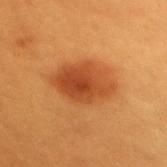Clinical impression:
The lesion was photographed on a routine skin check and not biopsied; there is no pathology result.
Background:
A lesion tile, about 15 mm wide, cut from a 3D total-body photograph. The patient is a female in their 30s. Captured under cross-polarized illumination. From the mid back. Automated tile analysis of the lesion measured a lesion color around L≈45 a*≈29 b*≈41 in CIELAB, about 11 CIELAB-L* units darker than the surrounding skin, and a normalized border contrast of about 8.5. And it measured an automated nevus-likeness rating near 100 out of 100 and a lesion-detection confidence of about 100/100. Longest diameter approximately 6 mm.